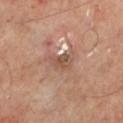Imaged during a routine full-body skin examination; the lesion was not biopsied and no histopathology is available. A roughly 15 mm field-of-view crop from a total-body skin photograph. Captured under cross-polarized illumination. Approximately 3.5 mm at its widest. The total-body-photography lesion software estimated an area of roughly 5 mm², an outline eccentricity of about 0.85 (0 = round, 1 = elongated), and a symmetry-axis asymmetry near 0.3. And it measured a color-variation rating of about 4/10 and a peripheral color-asymmetry measure near 1. The analysis additionally found a nevus-likeness score of about 0/100 and a detector confidence of about 90 out of 100 that the crop contains a lesion. Located on the left lower leg. A male patient roughly 50 years of age.